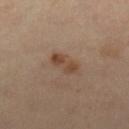Captured during whole-body skin photography for melanoma surveillance; the lesion was not biopsied. A 15 mm close-up extracted from a 3D total-body photography capture. The lesion-visualizer software estimated border irregularity of about 3.5 on a 0–10 scale and radial color variation of about 1. And it measured a nevus-likeness score of about 45/100 and a lesion-detection confidence of about 100/100. About 4 mm across. The subject is a female roughly 40 years of age. The lesion is located on the left leg. This is a cross-polarized tile.